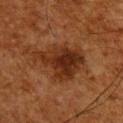Recorded during total-body skin imaging; not selected for excision or biopsy. The lesion's longest dimension is about 7 mm. The tile uses cross-polarized illumination. Automated tile analysis of the lesion measured a lesion area of about 20 mm². The software also gave a mean CIELAB color near L≈25 a*≈20 b*≈27, about 9 CIELAB-L* units darker than the surrounding skin, and a normalized lesion–skin contrast near 9.5. The analysis additionally found an automated nevus-likeness rating near 70 out of 100 and lesion-presence confidence of about 100/100. The lesion is located on the upper back. A male subject, about 65 years old. A 15 mm close-up extracted from a 3D total-body photography capture.